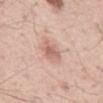The lesion was photographed on a routine skin check and not biopsied; there is no pathology result. This image is a 15 mm lesion crop taken from a total-body photograph. The lesion's longest dimension is about 3 mm. Located on the abdomen. A male subject, approximately 55 years of age.